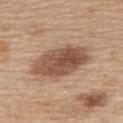The lesion was photographed on a routine skin check and not biopsied; there is no pathology result.
Located on the upper back.
The lesion's longest dimension is about 7.5 mm.
A female patient, roughly 65 years of age.
A roughly 15 mm field-of-view crop from a total-body skin photograph.
The tile uses white-light illumination.
The lesion-visualizer software estimated a lesion area of about 21 mm², an eccentricity of roughly 0.85, and a shape-asymmetry score of about 0.15 (0 = symmetric). The software also gave a border-irregularity rating of about 2/10, a within-lesion color-variation index near 5.5/10, and a peripheral color-asymmetry measure near 2. The software also gave a classifier nevus-likeness of about 15/100 and a detector confidence of about 100 out of 100 that the crop contains a lesion.